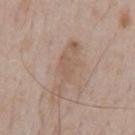No biopsy was performed on this lesion — it was imaged during a full skin examination and was not determined to be concerning. On the chest. A male patient, aged approximately 65. A 15 mm crop from a total-body photograph taken for skin-cancer surveillance.